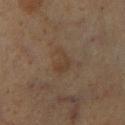The lesion is located on the right lower leg. This image is a 15 mm lesion crop taken from a total-body photograph. The recorded lesion diameter is about 3.5 mm. A male patient, aged approximately 60. The tile uses cross-polarized illumination.